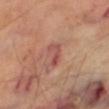The lesion was photographed on a routine skin check and not biopsied; there is no pathology result. Imaged with cross-polarized lighting. From the leg. The subject is a male approximately 70 years of age. Cropped from a total-body skin-imaging series; the visible field is about 15 mm.